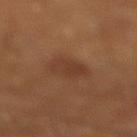Recorded during total-body skin imaging; not selected for excision or biopsy.
The recorded lesion diameter is about 3.5 mm.
The tile uses cross-polarized illumination.
The lesion is on the leg.
The subject is a male about 60 years old.
A 15 mm close-up extracted from a 3D total-body photography capture.